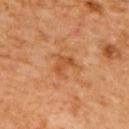image-analysis metrics = a footprint of about 4 mm²; a lesion color around L≈49 a*≈26 b*≈40 in CIELAB and a normalized lesion–skin contrast near 6; a border-irregularity index near 4/10, a color-variation rating of about 2.5/10, and peripheral color asymmetry of about 1; a classifier nevus-likeness of about 0/100 and a lesion-detection confidence of about 100/100
tile lighting = cross-polarized
diameter = ≈2.5 mm
site = the upper back
patient = male, aged approximately 65
image = ~15 mm crop, total-body skin-cancer survey